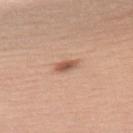notes: imaged on a skin check; not biopsied
subject: female, aged around 60
automated metrics: about 12 CIELAB-L* units darker than the surrounding skin and a lesion-to-skin contrast of about 8 (normalized; higher = more distinct)
diameter: ≈3 mm
body site: the upper back
image: total-body-photography crop, ~15 mm field of view
illumination: white-light illumination